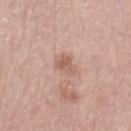Recorded during total-body skin imaging; not selected for excision or biopsy. The lesion is on the right thigh. Captured under white-light illumination. A roughly 15 mm field-of-view crop from a total-body skin photograph. A female patient in their mid-60s. The lesion's longest dimension is about 2.5 mm. Automated tile analysis of the lesion measured an average lesion color of about L≈59 a*≈20 b*≈26 (CIELAB) and a lesion-to-skin contrast of about 6.5 (normalized; higher = more distinct). It also reported border irregularity of about 3 on a 0–10 scale and a peripheral color-asymmetry measure near 1.5. And it measured an automated nevus-likeness rating near 5 out of 100 and a detector confidence of about 100 out of 100 that the crop contains a lesion.